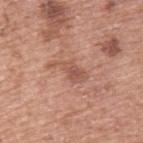biopsy_status: not biopsied; imaged during a skin examination
patient:
  sex: male
  age_approx: 55
site: upper back
image:
  source: total-body photography crop
  field_of_view_mm: 15
automated_metrics:
  area_mm2_approx: 5.5
  shape_asymmetry: 0.55
  vs_skin_darker_L: 9.0
  vs_skin_contrast_norm: 6.5
  border_irregularity_0_10: 6.5
  color_variation_0_10: 2.5
  peripheral_color_asymmetry: 1.0